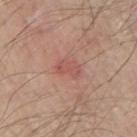follow-up=total-body-photography surveillance lesion; no biopsy | location=the right upper arm | illumination=white-light illumination | subject=male, roughly 65 years of age | image source=~15 mm crop, total-body skin-cancer survey.